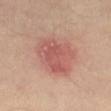Clinical impression: No biopsy was performed on this lesion — it was imaged during a full skin examination and was not determined to be concerning. Clinical summary: The subject is a male in their mid-50s. Approximately 6 mm at its widest. Captured under cross-polarized illumination. On the left thigh. This image is a 15 mm lesion crop taken from a total-body photograph.